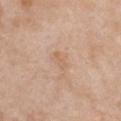Q: How was this image acquired?
A: 15 mm crop, total-body photography
Q: What are the patient's age and sex?
A: female, aged 38 to 42
Q: Lesion location?
A: the chest
Q: How was the tile lit?
A: white-light illumination